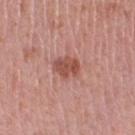Assessment: Recorded during total-body skin imaging; not selected for excision or biopsy. Clinical summary: The lesion-visualizer software estimated a lesion area of about 6 mm². The software also gave a mean CIELAB color near L≈51 a*≈26 b*≈27 and a normalized border contrast of about 8. And it measured a classifier nevus-likeness of about 70/100 and lesion-presence confidence of about 100/100. A 15 mm close-up tile from a total-body photography series done for melanoma screening. This is a white-light tile. Approximately 3 mm at its widest. On the right thigh. A female patient, aged around 40.